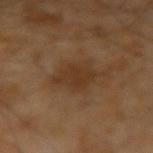Notes:
– notes · catalogued during a skin exam; not biopsied
– patient · male, aged around 65
– acquisition · 15 mm crop, total-body photography
– TBP lesion metrics · a footprint of about 9 mm², a shape eccentricity near 0.65, and a symmetry-axis asymmetry near 0.3